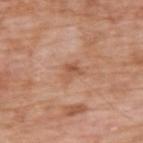Recorded during total-body skin imaging; not selected for excision or biopsy. Approximately 2.5 mm at its widest. The lesion is located on the upper back. The patient is a male roughly 60 years of age. Cropped from a whole-body photographic skin survey; the tile spans about 15 mm. This is a white-light tile. An algorithmic analysis of the crop reported a lesion area of about 3.5 mm², an eccentricity of roughly 0.7, and a symmetry-axis asymmetry near 0.4. It also reported a mean CIELAB color near L≈55 a*≈23 b*≈33 and a normalized lesion–skin contrast near 6.